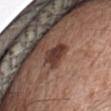Q: Was a biopsy performed?
A: catalogued during a skin exam; not biopsied
Q: What kind of image is this?
A: 15 mm crop, total-body photography
Q: Who is the patient?
A: male, aged around 60
Q: Where on the body is the lesion?
A: the left forearm